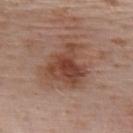Assessment: Imaged during a routine full-body skin examination; the lesion was not biopsied and no histopathology is available. Acquisition and patient details: The lesion's longest dimension is about 5.5 mm. From the upper back. A male patient in their 40s. This image is a 15 mm lesion crop taken from a total-body photograph.